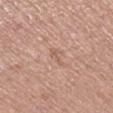Clinical impression:
The lesion was photographed on a routine skin check and not biopsied; there is no pathology result.
Image and clinical context:
A female patient, aged around 50. Cropped from a total-body skin-imaging series; the visible field is about 15 mm. On the right lower leg. An algorithmic analysis of the crop reported a lesion–skin lightness drop of about 7. And it measured a border-irregularity rating of about 5.5/10, a color-variation rating of about 0/10, and radial color variation of about 0. Captured under white-light illumination. The recorded lesion diameter is about 3 mm.